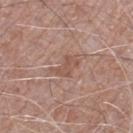Case summary:
* notes · total-body-photography surveillance lesion; no biopsy
* image source · 15 mm crop, total-body photography
* lesion size · ≈3.5 mm
* TBP lesion metrics · an area of roughly 5 mm², a shape eccentricity near 0.8, and a shape-asymmetry score of about 0.45 (0 = symmetric)
* lighting · white-light illumination
* patient · male, about 75 years old
* site · the right lower leg